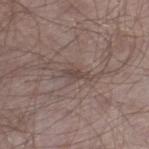The patient is a male aged approximately 65.
The lesion is on the right lower leg.
Cropped from a total-body skin-imaging series; the visible field is about 15 mm.
The tile uses white-light illumination.
About 2.5 mm across.